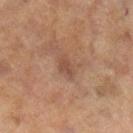  lighting: cross-polarized
  image:
    source: total-body photography crop
    field_of_view_mm: 15
  patient:
    sex: female
    age_approx: 60
  lesion_size:
    long_diameter_mm_approx: 3.0
  site: right lower leg